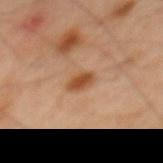{"site": "back", "lighting": "cross-polarized", "lesion_size": {"long_diameter_mm_approx": 3.0}, "image": {"source": "total-body photography crop", "field_of_view_mm": 15}, "patient": {"sex": "male", "age_approx": 45}, "automated_metrics": {"nevus_likeness_0_100": 85}}